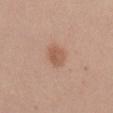No biopsy was performed on this lesion — it was imaged during a full skin examination and was not determined to be concerning. The tile uses white-light illumination. From the mid back. The recorded lesion diameter is about 2.5 mm. A roughly 15 mm field-of-view crop from a total-body skin photograph. The total-body-photography lesion software estimated a lesion area of about 4.5 mm², an eccentricity of roughly 0.65, and a shape-asymmetry score of about 0.2 (0 = symmetric). And it measured an average lesion color of about L≈56 a*≈21 b*≈31 (CIELAB), a lesion–skin lightness drop of about 9, and a normalized border contrast of about 6.5. It also reported a border-irregularity rating of about 1.5/10, a color-variation rating of about 2.5/10, and peripheral color asymmetry of about 1. It also reported an automated nevus-likeness rating near 85 out of 100. A female patient, aged 38 to 42.